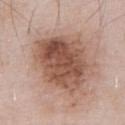| field | value |
|---|---|
| notes | catalogued during a skin exam; not biopsied |
| site | the abdomen |
| subject | male, about 55 years old |
| acquisition | ~15 mm tile from a whole-body skin photo |